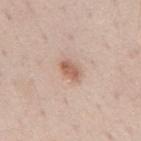No biopsy was performed on this lesion — it was imaged during a full skin examination and was not determined to be concerning.
A close-up tile cropped from a whole-body skin photograph, about 15 mm across.
On the back.
The patient is a male roughly 70 years of age.